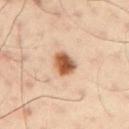Captured during whole-body skin photography for melanoma surveillance; the lesion was not biopsied.
The lesion is located on the left arm.
Cropped from a whole-body photographic skin survey; the tile spans about 15 mm.
A male patient, aged 48–52.
This is a cross-polarized tile.
Approximately 3.5 mm at its widest.